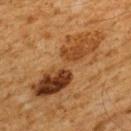follow-up=catalogued during a skin exam; not biopsied | patient=male, in their 60s | imaging modality=total-body-photography crop, ~15 mm field of view | anatomic site=the upper back.